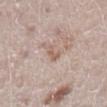Q: Was a biopsy performed?
A: imaged on a skin check; not biopsied
Q: Illumination type?
A: white-light
Q: Patient demographics?
A: female, roughly 50 years of age
Q: Where on the body is the lesion?
A: the leg
Q: What kind of image is this?
A: total-body-photography crop, ~15 mm field of view
Q: What is the lesion's diameter?
A: ≈3 mm